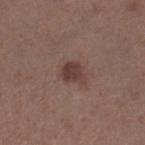{
  "biopsy_status": "not biopsied; imaged during a skin examination",
  "patient": {
    "sex": "female",
    "age_approx": 40
  },
  "automated_metrics": {
    "area_mm2_approx": 5.0,
    "eccentricity": 0.55,
    "shape_asymmetry": 0.25,
    "cielab_L": 38,
    "cielab_a": 19,
    "cielab_b": 21,
    "vs_skin_darker_L": 10.0,
    "vs_skin_contrast_norm": 8.5,
    "nevus_likeness_0_100": 75,
    "lesion_detection_confidence_0_100": 100
  },
  "site": "right lower leg",
  "image": {
    "source": "total-body photography crop",
    "field_of_view_mm": 15
  },
  "lesion_size": {
    "long_diameter_mm_approx": 2.5
  }
}